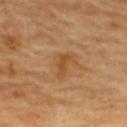workup: catalogued during a skin exam; not biopsied
lesion size: ~3 mm (longest diameter)
illumination: cross-polarized illumination
image: 15 mm crop, total-body photography
patient: female, roughly 60 years of age
body site: the upper back